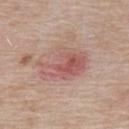Assessment: No biopsy was performed on this lesion — it was imaged during a full skin examination and was not determined to be concerning. Image and clinical context: The lesion is located on the upper back. A male patient, in their mid- to late 70s. A close-up tile cropped from a whole-body skin photograph, about 15 mm across.